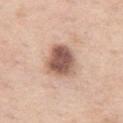Imaged during a routine full-body skin examination; the lesion was not biopsied and no histopathology is available.
Imaged with white-light lighting.
A female patient, aged around 55.
Located on the left thigh.
The lesion's longest dimension is about 4 mm.
The total-body-photography lesion software estimated a border-irregularity rating of about 1.5/10 and radial color variation of about 1.5.
Cropped from a whole-body photographic skin survey; the tile spans about 15 mm.